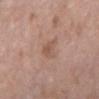Q: What is the anatomic site?
A: the chest
Q: What are the patient's age and sex?
A: female, aged around 75
Q: What kind of image is this?
A: total-body-photography crop, ~15 mm field of view